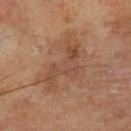Impression:
This lesion was catalogued during total-body skin photography and was not selected for biopsy.
Background:
About 7.5 mm across. A 15 mm crop from a total-body photograph taken for skin-cancer surveillance. The lesion is located on the leg. Automated tile analysis of the lesion measured an area of roughly 17 mm² and a shape-asymmetry score of about 0.6 (0 = symmetric). It also reported a nevus-likeness score of about 5/100 and lesion-presence confidence of about 100/100. A male subject about 65 years old.